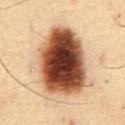This lesion was catalogued during total-body skin photography and was not selected for biopsy.
A close-up tile cropped from a whole-body skin photograph, about 15 mm across.
The lesion's longest dimension is about 8.5 mm.
The patient is a male aged 58–62.
The lesion is located on the abdomen.
The tile uses cross-polarized illumination.